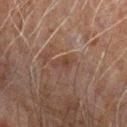| key | value |
|---|---|
| follow-up | catalogued during a skin exam; not biopsied |
| patient | male, about 60 years old |
| image-analysis metrics | an area of roughly 4 mm² and two-axis asymmetry of about 0.35; border irregularity of about 3.5 on a 0–10 scale, internal color variation of about 2 on a 0–10 scale, and a peripheral color-asymmetry measure near 0.5; a nevus-likeness score of about 0/100 |
| body site | the left forearm |
| diameter | ~3 mm (longest diameter) |
| illumination | cross-polarized illumination |
| imaging modality | ~15 mm crop, total-body skin-cancer survey |